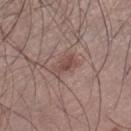{
  "biopsy_status": "not biopsied; imaged during a skin examination",
  "image": {
    "source": "total-body photography crop",
    "field_of_view_mm": 15
  },
  "site": "leg",
  "automated_metrics": {
    "area_mm2_approx": 4.0,
    "eccentricity": 0.9,
    "shape_asymmetry": 0.45,
    "cielab_L": 47,
    "cielab_a": 19,
    "cielab_b": 22,
    "vs_skin_darker_L": 8.0,
    "vs_skin_contrast_norm": 6.5,
    "border_irregularity_0_10": 5.0,
    "color_variation_0_10": 1.5
  },
  "lesion_size": {
    "long_diameter_mm_approx": 3.5
  },
  "patient": {
    "sex": "male",
    "age_approx": 35
  }
}